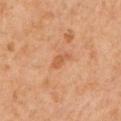notes=imaged on a skin check; not biopsied | TBP lesion metrics=an area of roughly 3 mm² and an eccentricity of roughly 0.9; an automated nevus-likeness rating near 0 out of 100 | imaging modality=~15 mm tile from a whole-body skin photo | patient=male, in their 60s | body site=the left upper arm | lighting=cross-polarized illumination.